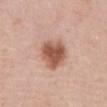Assessment: The lesion was photographed on a routine skin check and not biopsied; there is no pathology result. Context: The total-body-photography lesion software estimated a footprint of about 12 mm², an outline eccentricity of about 0.5 (0 = round, 1 = elongated), and two-axis asymmetry of about 0.25. It also reported a color-variation rating of about 4.5/10 and peripheral color asymmetry of about 1.5. A region of skin cropped from a whole-body photographic capture, roughly 15 mm wide. Imaged with white-light lighting. The lesion is on the abdomen. A female patient in their mid- to late 70s. Approximately 4 mm at its widest.